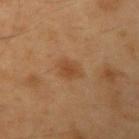| feature | finding |
|---|---|
| TBP lesion metrics | a lesion area of about 5 mm², an eccentricity of roughly 0.8, and a symmetry-axis asymmetry near 0.2 |
| subject | male, aged around 50 |
| anatomic site | the left upper arm |
| image | ~15 mm tile from a whole-body skin photo |